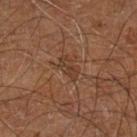Case summary:
- lesion diameter — about 3 mm
- location — the right leg
- automated lesion analysis — an area of roughly 3.5 mm², an eccentricity of roughly 0.9, and two-axis asymmetry of about 0.45
- lighting — cross-polarized
- imaging modality — ~15 mm tile from a whole-body skin photo
- patient — male, aged 58 to 62